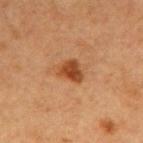Q: Was a biopsy performed?
A: imaged on a skin check; not biopsied
Q: What is the anatomic site?
A: the right upper arm
Q: How large is the lesion?
A: ~2.5 mm (longest diameter)
Q: What kind of image is this?
A: 15 mm crop, total-body photography
Q: What did automated image analysis measure?
A: an area of roughly 5 mm², an eccentricity of roughly 0.5, and two-axis asymmetry of about 0.35; a border-irregularity rating of about 3/10, a color-variation rating of about 2.5/10, and a peripheral color-asymmetry measure near 0.5; a classifier nevus-likeness of about 95/100
Q: Patient demographics?
A: female, aged approximately 40